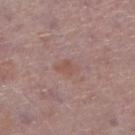Impression:
Part of a total-body skin-imaging series; this lesion was reviewed on a skin check and was not flagged for biopsy.
Clinical summary:
The tile uses white-light illumination. A male patient aged approximately 75. Cropped from a total-body skin-imaging series; the visible field is about 15 mm. The lesion is on the right lower leg. The lesion-visualizer software estimated an outline eccentricity of about 0.75 (0 = round, 1 = elongated) and two-axis asymmetry of about 0.25. The analysis additionally found a lesion color around L≈52 a*≈20 b*≈24 in CIELAB, about 5 CIELAB-L* units darker than the surrounding skin, and a normalized border contrast of about 5. The analysis additionally found border irregularity of about 2.5 on a 0–10 scale, internal color variation of about 1.5 on a 0–10 scale, and peripheral color asymmetry of about 0.5.